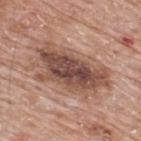The lesion was photographed on a routine skin check and not biopsied; there is no pathology result.
Cropped from a whole-body photographic skin survey; the tile spans about 15 mm.
The patient is a male roughly 65 years of age.
The lesion-visualizer software estimated a mean CIELAB color near L≈50 a*≈19 b*≈26, about 13 CIELAB-L* units darker than the surrounding skin, and a lesion-to-skin contrast of about 9.5 (normalized; higher = more distinct). The analysis additionally found border irregularity of about 5 on a 0–10 scale, a color-variation rating of about 7.5/10, and peripheral color asymmetry of about 2.5.
On the upper back.